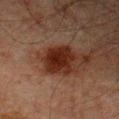Impression:
Recorded during total-body skin imaging; not selected for excision or biopsy.